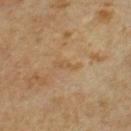A male patient, roughly 65 years of age. Located on the chest. The recorded lesion diameter is about 2.5 mm. A close-up tile cropped from a whole-body skin photograph, about 15 mm across. This is a cross-polarized tile. Automated tile analysis of the lesion measured a mean CIELAB color near L≈48 a*≈14 b*≈33. And it measured a nevus-likeness score of about 0/100 and a lesion-detection confidence of about 100/100.